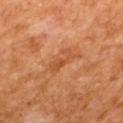biopsy_status: not biopsied; imaged during a skin examination
image:
  source: total-body photography crop
  field_of_view_mm: 15
patient:
  sex: male
  age_approx: 65
site: mid back
lesion_size:
  long_diameter_mm_approx: 3.5
lighting: cross-polarized